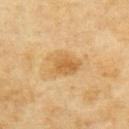{"biopsy_status": "not biopsied; imaged during a skin examination", "patient": {"sex": "female", "age_approx": 60}, "site": "upper back", "lighting": "cross-polarized", "automated_metrics": {"cielab_L": 57, "cielab_a": 17, "cielab_b": 41, "vs_skin_darker_L": 9.0, "vs_skin_contrast_norm": 6.5, "color_variation_0_10": 4.0, "peripheral_color_asymmetry": 1.5, "nevus_likeness_0_100": 35}, "image": {"source": "total-body photography crop", "field_of_view_mm": 15}}